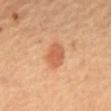Case summary:
* workup — no biopsy performed (imaged during a skin exam)
* illumination — cross-polarized
* anatomic site — the front of the torso
* image source — ~15 mm crop, total-body skin-cancer survey
* subject — female, aged 58–62
* diameter — about 3.5 mm